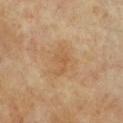Assessment: Recorded during total-body skin imaging; not selected for excision or biopsy. Clinical summary: Cropped from a whole-body photographic skin survey; the tile spans about 15 mm. The lesion-visualizer software estimated a shape eccentricity near 0.85 and two-axis asymmetry of about 0.35. The analysis additionally found a mean CIELAB color near L≈51 a*≈17 b*≈34, roughly 6 lightness units darker than nearby skin, and a normalized lesion–skin contrast near 5. It also reported an automated nevus-likeness rating near 0 out of 100 and lesion-presence confidence of about 100/100. Imaged with cross-polarized lighting. The patient is a female in their 60s. On the chest. Longest diameter approximately 3.5 mm.